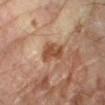* biopsy status · no biopsy performed (imaged during a skin exam)